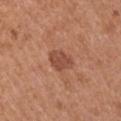notes = total-body-photography surveillance lesion; no biopsy | patient = male, in their mid- to late 50s | acquisition = 15 mm crop, total-body photography | automated metrics = a nevus-likeness score of about 25/100 and a lesion-detection confidence of about 100/100 | body site = the left upper arm | tile lighting = white-light illumination | diameter = about 3 mm.